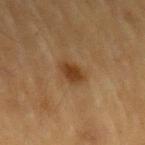Imaged during a routine full-body skin examination; the lesion was not biopsied and no histopathology is available.